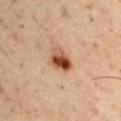Assessment:
This lesion was catalogued during total-body skin photography and was not selected for biopsy.
Acquisition and patient details:
An algorithmic analysis of the crop reported an average lesion color of about L≈48 a*≈23 b*≈33 (CIELAB), a lesion–skin lightness drop of about 16, and a normalized border contrast of about 11.5. It also reported a nevus-likeness score of about 100/100 and a lesion-detection confidence of about 100/100. A 15 mm close-up tile from a total-body photography series done for melanoma screening. A male patient, aged approximately 50. Captured under cross-polarized illumination. Located on the arm.